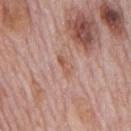- follow-up: imaged on a skin check; not biopsied
- acquisition: ~15 mm tile from a whole-body skin photo
- tile lighting: white-light illumination
- lesion diameter: ≈2.5 mm
- site: the mid back
- patient: male, approximately 75 years of age